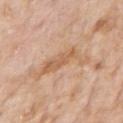{"biopsy_status": "not biopsied; imaged during a skin examination", "site": "right upper arm", "patient": {"sex": "male", "age_approx": 75}, "automated_metrics": {"cielab_L": 61, "cielab_a": 20, "cielab_b": 35, "vs_skin_darker_L": 8.0, "nevus_likeness_0_100": 0}, "lesion_size": {"long_diameter_mm_approx": 6.5}, "image": {"source": "total-body photography crop", "field_of_view_mm": 15}, "lighting": "white-light"}